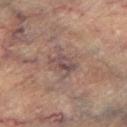The lesion was photographed on a routine skin check and not biopsied; there is no pathology result. A 15 mm crop from a total-body photograph taken for skin-cancer surveillance. A male subject aged approximately 75. Captured under cross-polarized illumination. On the left lower leg. The recorded lesion diameter is about 3.5 mm.